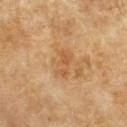This lesion was catalogued during total-body skin photography and was not selected for biopsy.
Cropped from a total-body skin-imaging series; the visible field is about 15 mm.
The lesion is located on the left forearm.
A female patient aged around 70.